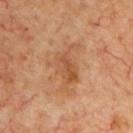Q: Lesion location?
A: the chest
Q: How large is the lesion?
A: about 5.5 mm
Q: What are the patient's age and sex?
A: male, aged 68 to 72
Q: What kind of image is this?
A: 15 mm crop, total-body photography